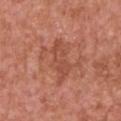| key | value |
|---|---|
| biopsy status | catalogued during a skin exam; not biopsied |
| acquisition | ~15 mm crop, total-body skin-cancer survey |
| automated lesion analysis | a lesion color around L≈50 a*≈27 b*≈32 in CIELAB, roughly 7 lightness units darker than nearby skin, and a normalized border contrast of about 5.5; a border-irregularity index near 5/10, a within-lesion color-variation index near 2/10, and radial color variation of about 0.5 |
| size | about 5 mm |
| illumination | white-light |
| patient | male, approximately 65 years of age |
| location | the chest |